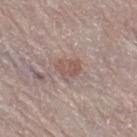biopsy status = no biopsy performed (imaged during a skin exam)
body site = the leg
subject = female, aged approximately 65
image = total-body-photography crop, ~15 mm field of view